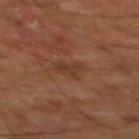biopsy status=imaged on a skin check; not biopsied | location=the chest | automated lesion analysis=an average lesion color of about L≈32 a*≈19 b*≈26 (CIELAB); border irregularity of about 4 on a 0–10 scale, internal color variation of about 2.5 on a 0–10 scale, and peripheral color asymmetry of about 1 | subject=male, aged around 60 | lesion size=~2.5 mm (longest diameter) | illumination=cross-polarized | image=~15 mm crop, total-body skin-cancer survey.